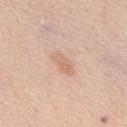The lesion was tiled from a total-body skin photograph and was not biopsied. Approximately 3.5 mm at its widest. Automated image analysis of the tile measured an area of roughly 5 mm², an eccentricity of roughly 0.9, and a shape-asymmetry score of about 0.25 (0 = symmetric). And it measured a mean CIELAB color near L≈67 a*≈19 b*≈31, about 7 CIELAB-L* units darker than the surrounding skin, and a lesion-to-skin contrast of about 5.5 (normalized; higher = more distinct). And it measured lesion-presence confidence of about 100/100. The subject is a male about 60 years old. A lesion tile, about 15 mm wide, cut from a 3D total-body photograph. The lesion is on the mid back. This is a white-light tile.